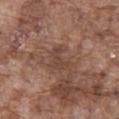Q: Was a biopsy performed?
A: no biopsy performed (imaged during a skin exam)